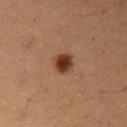biopsy_status: not biopsied; imaged during a skin examination
patient:
  sex: female
  age_approx: 35
site: right upper arm
lighting: cross-polarized
lesion_size:
  long_diameter_mm_approx: 2.5
image:
  source: total-body photography crop
  field_of_view_mm: 15